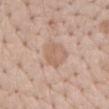Impression:
No biopsy was performed on this lesion — it was imaged during a full skin examination and was not determined to be concerning.
Acquisition and patient details:
Imaged with white-light lighting. Approximately 4.5 mm at its widest. From the lower back. A female patient aged 73–77. A 15 mm close-up extracted from a 3D total-body photography capture.